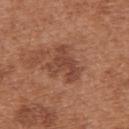Part of a total-body skin-imaging series; this lesion was reviewed on a skin check and was not flagged for biopsy.
The patient is a female about 40 years old.
An algorithmic analysis of the crop reported border irregularity of about 6 on a 0–10 scale, a color-variation rating of about 3/10, and a peripheral color-asymmetry measure near 1.
Approximately 4.5 mm at its widest.
The tile uses white-light illumination.
This image is a 15 mm lesion crop taken from a total-body photograph.
The lesion is located on the upper back.